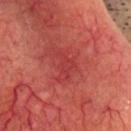Notes:
- follow-up · imaged on a skin check; not biopsied
- size · ≈3.5 mm
- automated lesion analysis · a footprint of about 5 mm² and a shape eccentricity near 0.85; a mean CIELAB color near L≈42 a*≈40 b*≈29, a lesion–skin lightness drop of about 6, and a normalized border contrast of about 4.5
- lighting · cross-polarized illumination
- anatomic site · the head or neck
- subject · male, aged 63 to 67
- acquisition · 15 mm crop, total-body photography